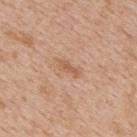workup: catalogued during a skin exam; not biopsied | illumination: white-light | lesion size: ~2.5 mm (longest diameter) | location: the upper back | subject: male, aged 63–67 | TBP lesion metrics: a lesion area of about 2 mm², an eccentricity of roughly 0.95, and two-axis asymmetry of about 0.45; an average lesion color of about L≈58 a*≈21 b*≈34 (CIELAB), about 8 CIELAB-L* units darker than the surrounding skin, and a lesion-to-skin contrast of about 6.5 (normalized; higher = more distinct); a border-irregularity index near 4.5/10, a within-lesion color-variation index near 0/10, and peripheral color asymmetry of about 0 | acquisition: 15 mm crop, total-body photography.